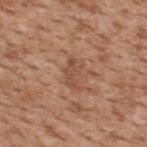notes=imaged on a skin check; not biopsied | lighting=white-light | lesion size=about 3.5 mm | location=the upper back | acquisition=~15 mm crop, total-body skin-cancer survey | subject=male, approximately 65 years of age.